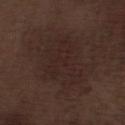| feature | finding |
|---|---|
| follow-up | catalogued during a skin exam; not biopsied |
| patient | male, aged 68 to 72 |
| illumination | white-light |
| image source | 15 mm crop, total-body photography |
| size | about 6.5 mm |
| location | the left lower leg |